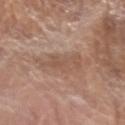{
  "biopsy_status": "not biopsied; imaged during a skin examination",
  "lesion_size": {
    "long_diameter_mm_approx": 5.0
  },
  "automated_metrics": {
    "eccentricity": 0.85,
    "border_irregularity_0_10": 5.5,
    "peripheral_color_asymmetry": 1.0,
    "nevus_likeness_0_100": 0,
    "lesion_detection_confidence_0_100": 90
  },
  "patient": {
    "sex": "female",
    "age_approx": 75
  },
  "image": {
    "source": "total-body photography crop",
    "field_of_view_mm": 15
  },
  "lighting": "white-light",
  "site": "left forearm"
}